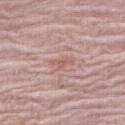Impression: Captured during whole-body skin photography for melanoma surveillance; the lesion was not biopsied.